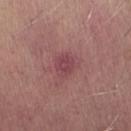Notes:
– follow-up · total-body-photography surveillance lesion; no biopsy
– tile lighting · white-light illumination
– subject · male, approximately 65 years of age
– site · the arm
– automated metrics · a footprint of about 4.5 mm² and a symmetry-axis asymmetry near 0.15; a mean CIELAB color near L≈44 a*≈27 b*≈17, roughly 8 lightness units darker than nearby skin, and a normalized lesion–skin contrast near 6.5; a border-irregularity rating of about 1.5/10, a color-variation rating of about 2/10, and radial color variation of about 0.5
– image · ~15 mm crop, total-body skin-cancer survey